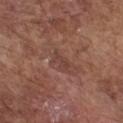This lesion was catalogued during total-body skin photography and was not selected for biopsy.
The lesion is located on the chest.
The patient is a male approximately 75 years of age.
A region of skin cropped from a whole-body photographic capture, roughly 15 mm wide.